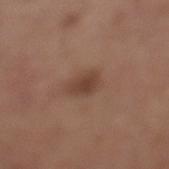| key | value |
|---|---|
| automated metrics | a footprint of about 5.5 mm² and a symmetry-axis asymmetry near 0.25; a lesion color around L≈42 a*≈19 b*≈27 in CIELAB and a lesion–skin lightness drop of about 9 |
| patient | female, roughly 65 years of age |
| location | the left lower leg |
| tile lighting | white-light |
| lesion size | about 3.5 mm |
| image source | ~15 mm tile from a whole-body skin photo |